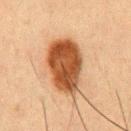patient: male, about 50 years old; site: the abdomen; image-analysis metrics: a lesion area of about 25 mm², an outline eccentricity of about 0.8 (0 = round, 1 = elongated), and a symmetry-axis asymmetry near 0.15; lesion diameter: about 7.5 mm; illumination: cross-polarized illumination; imaging modality: ~15 mm crop, total-body skin-cancer survey.